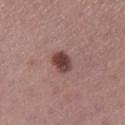This lesion was catalogued during total-body skin photography and was not selected for biopsy. The patient is a female aged around 60. The lesion is located on the leg. A roughly 15 mm field-of-view crop from a total-body skin photograph.